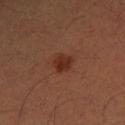Case summary:
– follow-up — no biopsy performed (imaged during a skin exam)
– patient — male, in their 50s
– location — the left lower leg
– image source — 15 mm crop, total-body photography